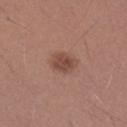The lesion was tiled from a total-body skin photograph and was not biopsied. A 15 mm close-up extracted from a 3D total-body photography capture. Located on the left forearm. Captured under white-light illumination. The recorded lesion diameter is about 3 mm. The patient is a male aged approximately 25.